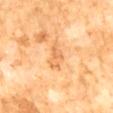Q: Is there a histopathology result?
A: catalogued during a skin exam; not biopsied
Q: How was this image acquired?
A: total-body-photography crop, ~15 mm field of view
Q: Lesion size?
A: ~3.5 mm (longest diameter)
Q: Where on the body is the lesion?
A: the abdomen
Q: Who is the patient?
A: male, roughly 60 years of age
Q: Illumination type?
A: cross-polarized
Q: Automated lesion metrics?
A: a footprint of about 5 mm² and a shape-asymmetry score of about 0.35 (0 = symmetric); a mean CIELAB color near L≈68 a*≈24 b*≈45 and a lesion-to-skin contrast of about 5.5 (normalized; higher = more distinct); border irregularity of about 4 on a 0–10 scale and radial color variation of about 0.5; an automated nevus-likeness rating near 0 out of 100 and a detector confidence of about 100 out of 100 that the crop contains a lesion